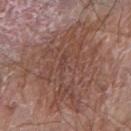This lesion was catalogued during total-body skin photography and was not selected for biopsy. The lesion is located on the left arm. The tile uses white-light illumination. A 15 mm close-up tile from a total-body photography series done for melanoma screening. The total-body-photography lesion software estimated a border-irregularity index near 9/10 and peripheral color asymmetry of about 1.5. And it measured lesion-presence confidence of about 100/100. A male subject roughly 80 years of age. Approximately 11 mm at its widest.